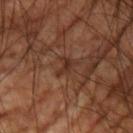This lesion was catalogued during total-body skin photography and was not selected for biopsy. Cropped from a total-body skin-imaging series; the visible field is about 15 mm. The lesion is located on the arm. Imaged with cross-polarized lighting. A male subject aged approximately 60. Measured at roughly 2.5 mm in maximum diameter.